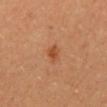The lesion was tiled from a total-body skin photograph and was not biopsied. Approximately 2 mm at its widest. Cropped from a total-body skin-imaging series; the visible field is about 15 mm. On the mid back. A female patient about 30 years old. Captured under cross-polarized illumination. The lesion-visualizer software estimated an average lesion color of about L≈50 a*≈28 b*≈38 (CIELAB). The software also gave a border-irregularity index near 2/10, a color-variation rating of about 2/10, and peripheral color asymmetry of about 0.5.